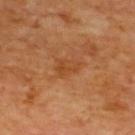Q: Was a biopsy performed?
A: imaged on a skin check; not biopsied
Q: What kind of image is this?
A: ~15 mm crop, total-body skin-cancer survey
Q: How large is the lesion?
A: ~3 mm (longest diameter)
Q: How was the tile lit?
A: cross-polarized illumination
Q: What is the anatomic site?
A: the upper back
Q: Who is the patient?
A: male, aged 58 to 62
Q: What did automated image analysis measure?
A: two-axis asymmetry of about 0.3; an automated nevus-likeness rating near 0 out of 100 and a detector confidence of about 100 out of 100 that the crop contains a lesion